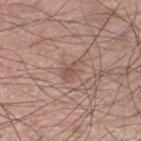follow-up: total-body-photography surveillance lesion; no biopsy | subject: male, about 60 years old | anatomic site: the leg | image source: 15 mm crop, total-body photography.